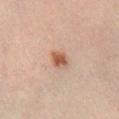Impression:
Imaged during a routine full-body skin examination; the lesion was not biopsied and no histopathology is available.
Clinical summary:
The tile uses cross-polarized illumination. Longest diameter approximately 2.5 mm. From the right lower leg. The lesion-visualizer software estimated a border-irregularity rating of about 2.5/10, internal color variation of about 3.5 on a 0–10 scale, and peripheral color asymmetry of about 1. And it measured a nevus-likeness score of about 95/100. A 15 mm close-up extracted from a 3D total-body photography capture. A male subject aged approximately 65.